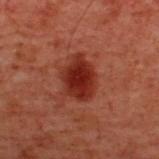<case>
  <biopsy_status>not biopsied; imaged during a skin examination</biopsy_status>
  <image>
    <source>total-body photography crop</source>
    <field_of_view_mm>15</field_of_view_mm>
  </image>
  <lesion_size>
    <long_diameter_mm_approx>4.5</long_diameter_mm_approx>
  </lesion_size>
  <site>upper back</site>
  <patient>
    <sex>male</sex>
    <age_approx>60</age_approx>
  </patient>
</case>